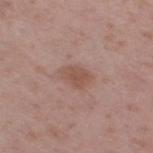* workup: catalogued during a skin exam; not biopsied
* anatomic site: the leg
* image source: total-body-photography crop, ~15 mm field of view
* patient: female, in their mid-50s